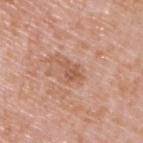Assessment: Captured during whole-body skin photography for melanoma surveillance; the lesion was not biopsied. Background: On the upper back. A male patient, aged 48 to 52. Automated tile analysis of the lesion measured a lesion–skin lightness drop of about 9 and a lesion-to-skin contrast of about 6 (normalized; higher = more distinct). A 15 mm close-up extracted from a 3D total-body photography capture. About 3 mm across.